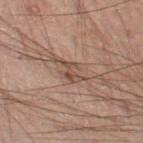Findings:
• workup: total-body-photography surveillance lesion; no biopsy
• patient: male, aged approximately 50
• site: the left thigh
• lesion diameter: ~3 mm (longest diameter)
• image source: ~15 mm tile from a whole-body skin photo